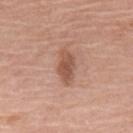workup: catalogued during a skin exam; not biopsied
lighting: white-light
image-analysis metrics: an area of roughly 6 mm², an eccentricity of roughly 0.85, and a symmetry-axis asymmetry near 0.25; border irregularity of about 3 on a 0–10 scale, internal color variation of about 2 on a 0–10 scale, and a peripheral color-asymmetry measure near 0.5; an automated nevus-likeness rating near 75 out of 100
subject: female, approximately 70 years of age
image source: 15 mm crop, total-body photography
location: the upper back